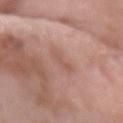Clinical impression: Recorded during total-body skin imaging; not selected for excision or biopsy. Background: A 15 mm close-up tile from a total-body photography series done for melanoma screening. A female subject, approximately 60 years of age. Captured under white-light illumination. The lesion is on the left forearm. The total-body-photography lesion software estimated a lesion color around L≈56 a*≈21 b*≈26 in CIELAB. The lesion's longest dimension is about 2.5 mm.